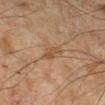workup — imaged on a skin check; not biopsied
acquisition — total-body-photography crop, ~15 mm field of view
illumination — cross-polarized illumination
patient — male, approximately 60 years of age
anatomic site — the left lower leg